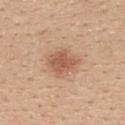Clinical impression: The lesion was photographed on a routine skin check and not biopsied; there is no pathology result. Background: A female subject, aged 43 to 47. Longest diameter approximately 4 mm. The lesion is on the upper back. This image is a 15 mm lesion crop taken from a total-body photograph. Imaged with white-light lighting.